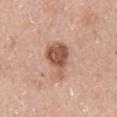{
  "biopsy_status": "not biopsied; imaged during a skin examination",
  "automated_metrics": {
    "area_mm2_approx": 10.0,
    "eccentricity": 0.8,
    "shape_asymmetry": 0.4,
    "cielab_L": 54,
    "cielab_a": 23,
    "cielab_b": 31,
    "vs_skin_contrast_norm": 9.5,
    "nevus_likeness_0_100": 60,
    "lesion_detection_confidence_0_100": 100
  },
  "lighting": "white-light",
  "site": "left upper arm",
  "image": {
    "source": "total-body photography crop",
    "field_of_view_mm": 15
  },
  "patient": {
    "sex": "female",
    "age_approx": 50
  },
  "lesion_size": {
    "long_diameter_mm_approx": 5.0
  }
}